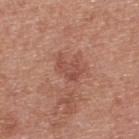Clinical impression: Imaged during a routine full-body skin examination; the lesion was not biopsied and no histopathology is available. Context: Measured at roughly 3.5 mm in maximum diameter. Located on the upper back. The subject is a male aged approximately 30. This is a white-light tile. A 15 mm close-up extracted from a 3D total-body photography capture.